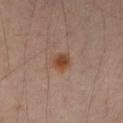On the left upper arm. A male subject aged 33 to 37. Imaged with cross-polarized lighting. Automated image analysis of the tile measured a lesion area of about 4.5 mm² and a shape eccentricity near 0.45. It also reported a lesion color around L≈43 a*≈20 b*≈29 in CIELAB and a lesion-to-skin contrast of about 9 (normalized; higher = more distinct). The analysis additionally found a lesion-detection confidence of about 100/100. This image is a 15 mm lesion crop taken from a total-body photograph.